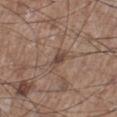follow-up: catalogued during a skin exam; not biopsied | lighting: white-light | lesion size: about 2.5 mm | imaging modality: ~15 mm tile from a whole-body skin photo | patient: male, approximately 65 years of age | TBP lesion metrics: a lesion area of about 4 mm², an outline eccentricity of about 0.8 (0 = round, 1 = elongated), and a shape-asymmetry score of about 0.3 (0 = symmetric); a border-irregularity rating of about 3/10, a color-variation rating of about 2.5/10, and radial color variation of about 0.5 | body site: the left lower leg.